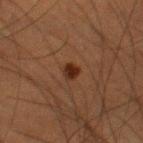The lesion was photographed on a routine skin check and not biopsied; there is no pathology result.